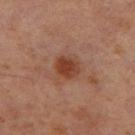workup = no biopsy performed (imaged during a skin exam)
site = the left thigh
lighting = cross-polarized
imaging modality = total-body-photography crop, ~15 mm field of view
size = ≈3 mm
patient = male, aged 58 to 62